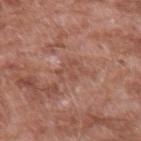• notes — catalogued during a skin exam; not biopsied
• lesion size — ≈3 mm
• lighting — white-light illumination
• subject — male, aged 58–62
• body site — the left upper arm
• image — ~15 mm crop, total-body skin-cancer survey
• automated lesion analysis — roughly 6 lightness units darker than nearby skin and a normalized lesion–skin contrast near 4.5; a classifier nevus-likeness of about 0/100 and a detector confidence of about 80 out of 100 that the crop contains a lesion